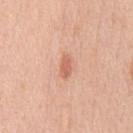| key | value |
|---|---|
| follow-up | total-body-photography surveillance lesion; no biopsy |
| lesion size | ≈2.5 mm |
| lighting | white-light illumination |
| location | the chest |
| subject | male, aged approximately 50 |
| image source | ~15 mm crop, total-body skin-cancer survey |
| automated metrics | a border-irregularity rating of about 2.5/10, internal color variation of about 1.5 on a 0–10 scale, and peripheral color asymmetry of about 0.5; an automated nevus-likeness rating near 50 out of 100 |